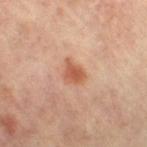illumination — cross-polarized illumination
subject — female, aged 63–67
diameter — ~3 mm (longest diameter)
TBP lesion metrics — a mean CIELAB color near L≈55 a*≈25 b*≈33, roughly 10 lightness units darker than nearby skin, and a lesion-to-skin contrast of about 7.5 (normalized; higher = more distinct); a border-irregularity rating of about 3/10, a within-lesion color-variation index near 2.5/10, and radial color variation of about 1; lesion-presence confidence of about 100/100
site — the left leg
acquisition — 15 mm crop, total-body photography